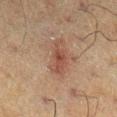Assessment: The lesion was photographed on a routine skin check and not biopsied; there is no pathology result. Background: From the right lower leg. A male subject, in their 60s. A close-up tile cropped from a whole-body skin photograph, about 15 mm across.